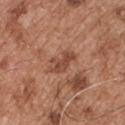The lesion was tiled from a total-body skin photograph and was not biopsied. On the chest. A male patient aged 53 to 57. The recorded lesion diameter is about 4 mm. Captured under white-light illumination. A close-up tile cropped from a whole-body skin photograph, about 15 mm across. An algorithmic analysis of the crop reported an average lesion color of about L≈47 a*≈24 b*≈30 (CIELAB), roughly 10 lightness units darker than nearby skin, and a normalized lesion–skin contrast near 7. It also reported border irregularity of about 4 on a 0–10 scale and peripheral color asymmetry of about 1.